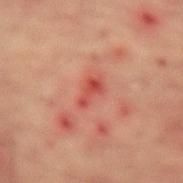<tbp_lesion>
  <biopsy_status>not biopsied; imaged during a skin examination</biopsy_status>
  <lighting>cross-polarized</lighting>
  <patient>
    <sex>male</sex>
    <age_approx>65</age_approx>
  </patient>
  <image>
    <source>total-body photography crop</source>
    <field_of_view_mm>15</field_of_view_mm>
  </image>
  <site>back</site>
</tbp_lesion>